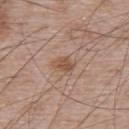Notes:
- follow-up: total-body-photography surveillance lesion; no biopsy
- lesion diameter: ~3 mm (longest diameter)
- automated metrics: a lesion color around L≈52 a*≈20 b*≈29 in CIELAB, roughly 9 lightness units darker than nearby skin, and a lesion-to-skin contrast of about 7 (normalized; higher = more distinct); a border-irregularity rating of about 2/10, internal color variation of about 2.5 on a 0–10 scale, and radial color variation of about 1; a nevus-likeness score of about 45/100 and a detector confidence of about 100 out of 100 that the crop contains a lesion
- imaging modality: 15 mm crop, total-body photography
- lighting: white-light illumination
- patient: male, about 65 years old
- anatomic site: the upper back